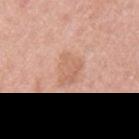This lesion was catalogued during total-body skin photography and was not selected for biopsy. The lesion is located on the left upper arm. A male subject aged 58 to 62. This image is a 15 mm lesion crop taken from a total-body photograph. Automated tile analysis of the lesion measured about 7 CIELAB-L* units darker than the surrounding skin and a lesion-to-skin contrast of about 5 (normalized; higher = more distinct). And it measured a classifier nevus-likeness of about 0/100 and lesion-presence confidence of about 100/100. The tile uses white-light illumination.